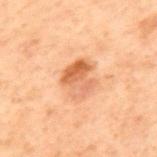Q: Is there a histopathology result?
A: catalogued during a skin exam; not biopsied
Q: What kind of image is this?
A: ~15 mm crop, total-body skin-cancer survey
Q: What did automated image analysis measure?
A: an average lesion color of about L≈49 a*≈21 b*≈32 (CIELAB) and roughly 10 lightness units darker than nearby skin; border irregularity of about 4 on a 0–10 scale, internal color variation of about 7.5 on a 0–10 scale, and a peripheral color-asymmetry measure near 2.5
Q: Lesion size?
A: about 3.5 mm
Q: How was the tile lit?
A: cross-polarized illumination
Q: Patient demographics?
A: male, aged 68–72
Q: Where on the body is the lesion?
A: the upper back